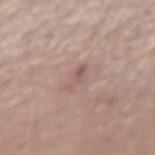Notes:
* biopsy status: catalogued during a skin exam; not biopsied
* site: the right forearm
* image: ~15 mm tile from a whole-body skin photo
* patient: male, aged 38–42